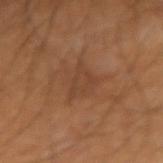The tile uses cross-polarized illumination.
Measured at roughly 4 mm in maximum diameter.
The lesion is located on the right forearm.
Cropped from a whole-body photographic skin survey; the tile spans about 15 mm.
An algorithmic analysis of the crop reported border irregularity of about 4.5 on a 0–10 scale and peripheral color asymmetry of about 0.5. The software also gave a nevus-likeness score of about 0/100 and a lesion-detection confidence of about 100/100.
A male patient, about 60 years old.